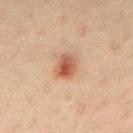The lesion was tiled from a total-body skin photograph and was not biopsied. The subject is a female approximately 40 years of age. Cropped from a whole-body photographic skin survey; the tile spans about 15 mm. The tile uses cross-polarized illumination. An algorithmic analysis of the crop reported an outline eccentricity of about 0.6 (0 = round, 1 = elongated) and a symmetry-axis asymmetry near 0.2. And it measured a nevus-likeness score of about 100/100 and lesion-presence confidence of about 100/100. The lesion is on the back.